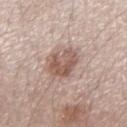The lesion was tiled from a total-body skin photograph and was not biopsied. Captured under white-light illumination. Located on the left forearm. An algorithmic analysis of the crop reported a footprint of about 9.5 mm² and a shape eccentricity near 0.5. It also reported border irregularity of about 3 on a 0–10 scale, a within-lesion color-variation index near 4.5/10, and radial color variation of about 1.5. A 15 mm close-up tile from a total-body photography series done for melanoma screening. A female subject, aged around 55. The lesion's longest dimension is about 4 mm.